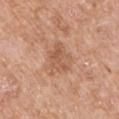The lesion is on the arm. A male patient aged approximately 60. A 15 mm close-up tile from a total-body photography series done for melanoma screening. About 4 mm across. Automated tile analysis of the lesion measured a mean CIELAB color near L≈57 a*≈22 b*≈32 and a normalized lesion–skin contrast near 5. And it measured a classifier nevus-likeness of about 0/100 and a detector confidence of about 100 out of 100 that the crop contains a lesion.